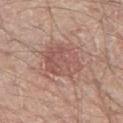{"biopsy_status": "not biopsied; imaged during a skin examination", "image": {"source": "total-body photography crop", "field_of_view_mm": 15}, "site": "left thigh", "patient": {"sex": "male", "age_approx": 60}}